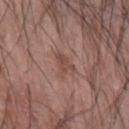Clinical impression: Part of a total-body skin-imaging series; this lesion was reviewed on a skin check and was not flagged for biopsy. Image and clinical context: An algorithmic analysis of the crop reported a lesion area of about 4 mm² and two-axis asymmetry of about 0.5. And it measured border irregularity of about 5.5 on a 0–10 scale and a peripheral color-asymmetry measure near 0.5. A male subject, aged approximately 70. Cropped from a total-body skin-imaging series; the visible field is about 15 mm. Measured at roughly 3 mm in maximum diameter. From the left forearm. Captured under white-light illumination.